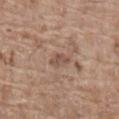Clinical summary:
Measured at roughly 3 mm in maximum diameter. A 15 mm close-up tile from a total-body photography series done for melanoma screening. This is a white-light tile. Automated image analysis of the tile measured a nevus-likeness score of about 0/100 and a detector confidence of about 95 out of 100 that the crop contains a lesion. From the lower back. A male patient, in their 80s.